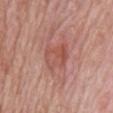Clinical summary: The tile uses white-light illumination. The patient is a male approximately 65 years of age. Located on the head or neck. A close-up tile cropped from a whole-body skin photograph, about 15 mm across.